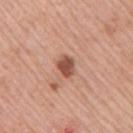No biopsy was performed on this lesion — it was imaged during a full skin examination and was not determined to be concerning.